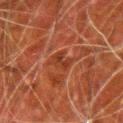Part of a total-body skin-imaging series; this lesion was reviewed on a skin check and was not flagged for biopsy. The lesion is on the right upper arm. A 15 mm close-up tile from a total-body photography series done for melanoma screening. A male patient, aged around 80. Longest diameter approximately 3 mm.